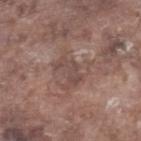| key | value |
|---|---|
| patient | male, approximately 75 years of age |
| lesion diameter | ≈3.5 mm |
| lighting | white-light |
| acquisition | ~15 mm crop, total-body skin-cancer survey |
| body site | the leg |